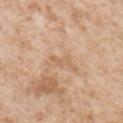biopsy status: no biopsy performed (imaged during a skin exam) | lighting: white-light illumination | subject: male, aged around 65 | anatomic site: the chest | automated metrics: a shape eccentricity near 0.9 and two-axis asymmetry of about 0.5; a lesion color around L≈62 a*≈18 b*≈34 in CIELAB, about 7 CIELAB-L* units darker than the surrounding skin, and a lesion-to-skin contrast of about 4.5 (normalized; higher = more distinct); internal color variation of about 0 on a 0–10 scale and a peripheral color-asymmetry measure near 0; a detector confidence of about 100 out of 100 that the crop contains a lesion | image source: ~15 mm crop, total-body skin-cancer survey | lesion size: about 3 mm.